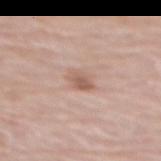{
  "site": "mid back",
  "automated_metrics": {
    "area_mm2_approx": 4.0,
    "eccentricity": 0.65,
    "shape_asymmetry": 0.2,
    "cielab_L": 59,
    "cielab_a": 19,
    "cielab_b": 27,
    "vs_skin_darker_L": 9.0,
    "vs_skin_contrast_norm": 6.5,
    "border_irregularity_0_10": 2.0,
    "peripheral_color_asymmetry": 1.0,
    "nevus_likeness_0_100": 60,
    "lesion_detection_confidence_0_100": 100
  },
  "image": {
    "source": "total-body photography crop",
    "field_of_view_mm": 15
  },
  "patient": {
    "sex": "female",
    "age_approx": 65
  }
}